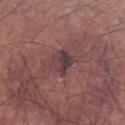{
  "biopsy_status": "not biopsied; imaged during a skin examination",
  "patient": {
    "sex": "male",
    "age_approx": 65
  },
  "automated_metrics": {
    "cielab_L": 38,
    "cielab_a": 18,
    "cielab_b": 13,
    "vs_skin_darker_L": 8.0,
    "border_irregularity_0_10": 2.5,
    "color_variation_0_10": 7.0,
    "peripheral_color_asymmetry": 2.5
  },
  "image": {
    "source": "total-body photography crop",
    "field_of_view_mm": 15
  },
  "site": "left forearm",
  "lighting": "white-light"
}